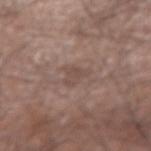notes: imaged on a skin check; not biopsied
body site: the right forearm
image source: 15 mm crop, total-body photography
patient: male, roughly 75 years of age
TBP lesion metrics: lesion-presence confidence of about 100/100